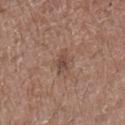Assessment: This lesion was catalogued during total-body skin photography and was not selected for biopsy. Clinical summary: Automated image analysis of the tile measured a lesion area of about 3.5 mm², an eccentricity of roughly 0.8, and two-axis asymmetry of about 0.3. The software also gave a lesion color around L≈46 a*≈18 b*≈24 in CIELAB and a normalized border contrast of about 6.5. And it measured a border-irregularity rating of about 3/10, internal color variation of about 2 on a 0–10 scale, and radial color variation of about 0.5. The analysis additionally found a classifier nevus-likeness of about 0/100 and lesion-presence confidence of about 100/100. A roughly 15 mm field-of-view crop from a total-body skin photograph. Measured at roughly 3 mm in maximum diameter. The tile uses white-light illumination. A male subject aged approximately 50. From the left forearm.